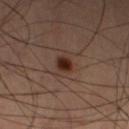follow-up = catalogued during a skin exam; not biopsied
subject = male, roughly 65 years of age
location = the left lower leg
acquisition = ~15 mm tile from a whole-body skin photo